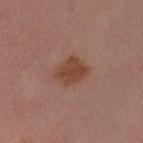Case summary:
– notes — catalogued during a skin exam; not biopsied
– location — the left forearm
– image source — ~15 mm crop, total-body skin-cancer survey
– patient — male, approximately 30 years of age